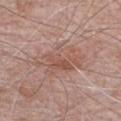<tbp_lesion>
  <biopsy_status>not biopsied; imaged during a skin examination</biopsy_status>
  <site>chest</site>
  <image>
    <source>total-body photography crop</source>
    <field_of_view_mm>15</field_of_view_mm>
  </image>
  <lighting>white-light</lighting>
  <automated_metrics>
    <area_mm2_approx>18.0</area_mm2_approx>
  </automated_metrics>
  <lesion_size>
    <long_diameter_mm_approx>6.5</long_diameter_mm_approx>
  </lesion_size>
  <patient>
    <sex>male</sex>
    <age_approx>70</age_approx>
  </patient>
</tbp_lesion>